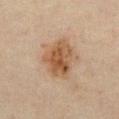On the chest. Imaged with cross-polarized lighting. The patient is a male aged 43–47. Cropped from a total-body skin-imaging series; the visible field is about 15 mm. About 4.5 mm across.